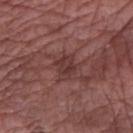– follow-up — catalogued during a skin exam; not biopsied
– image — total-body-photography crop, ~15 mm field of view
– site — the right forearm
– subject — male, aged 53–57
– lesion diameter — ~3 mm (longest diameter)